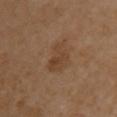– workup · imaged on a skin check; not biopsied
– lighting · cross-polarized illumination
– subject · female, roughly 70 years of age
– location · the chest
– image source · total-body-photography crop, ~15 mm field of view